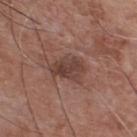Context: A 15 mm close-up extracted from a 3D total-body photography capture. A male subject about 75 years old. On the chest. Imaged with white-light lighting. The recorded lesion diameter is about 4 mm.